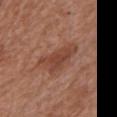| key | value |
|---|---|
| workup | no biopsy performed (imaged during a skin exam) |
| patient | female, aged around 75 |
| lighting | white-light illumination |
| lesion diameter | about 5 mm |
| acquisition | ~15 mm crop, total-body skin-cancer survey |
| anatomic site | the chest |
| automated metrics | an area of roughly 10 mm²; a lesion color around L≈44 a*≈23 b*≈29 in CIELAB, about 8 CIELAB-L* units darker than the surrounding skin, and a normalized lesion–skin contrast near 6.5; border irregularity of about 4 on a 0–10 scale and internal color variation of about 3 on a 0–10 scale; a nevus-likeness score of about 40/100 and a detector confidence of about 100 out of 100 that the crop contains a lesion |